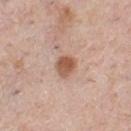Q: Was a biopsy performed?
A: no biopsy performed (imaged during a skin exam)
Q: What lighting was used for the tile?
A: white-light
Q: What is the imaging modality?
A: 15 mm crop, total-body photography
Q: What is the anatomic site?
A: the chest
Q: What did automated image analysis measure?
A: a shape eccentricity near 0.5 and a shape-asymmetry score of about 0.2 (0 = symmetric); a border-irregularity rating of about 1.5/10, a within-lesion color-variation index near 3/10, and peripheral color asymmetry of about 1
Q: Patient demographics?
A: male, approximately 40 years of age